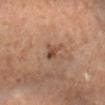Recorded during total-body skin imaging; not selected for excision or biopsy. The lesion is on the arm. This is a cross-polarized tile. The recorded lesion diameter is about 2 mm. The subject is a female in their mid- to late 50s. A 15 mm close-up extracted from a 3D total-body photography capture.